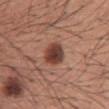Q: Was this lesion biopsied?
A: total-body-photography surveillance lesion; no biopsy
Q: How was this image acquired?
A: total-body-photography crop, ~15 mm field of view
Q: Where on the body is the lesion?
A: the back
Q: Patient demographics?
A: male, aged approximately 45